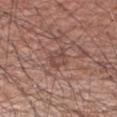biopsy_status: not biopsied; imaged during a skin examination
site: right upper arm
patient:
  sex: male
  age_approx: 60
lighting: white-light
image:
  source: total-body photography crop
  field_of_view_mm: 15